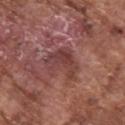follow-up: total-body-photography surveillance lesion; no biopsy | acquisition: ~15 mm tile from a whole-body skin photo | automated lesion analysis: border irregularity of about 6.5 on a 0–10 scale and a peripheral color-asymmetry measure near 1.5 | tile lighting: white-light illumination | patient: male, approximately 75 years of age | lesion size: about 4.5 mm | anatomic site: the right upper arm.